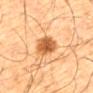Impression: Part of a total-body skin-imaging series; this lesion was reviewed on a skin check and was not flagged for biopsy. Acquisition and patient details: The lesion's longest dimension is about 5 mm. Cropped from a total-body skin-imaging series; the visible field is about 15 mm. Imaged with cross-polarized lighting. On the mid back. The patient is a male about 65 years old.